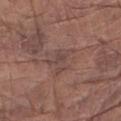{"biopsy_status": "not biopsied; imaged during a skin examination", "automated_metrics": {"area_mm2_approx": 6.0, "eccentricity": 0.65, "border_irregularity_0_10": 6.0, "color_variation_0_10": 2.0, "peripheral_color_asymmetry": 0.5, "lesion_detection_confidence_0_100": 65}, "image": {"source": "total-body photography crop", "field_of_view_mm": 15}, "patient": {"sex": "male", "age_approx": 80}, "lighting": "white-light", "site": "right upper arm", "lesion_size": {"long_diameter_mm_approx": 3.5}}